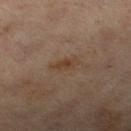This lesion was catalogued during total-body skin photography and was not selected for biopsy.
The lesion-visualizer software estimated a lesion color around L≈37 a*≈15 b*≈27 in CIELAB, a lesion–skin lightness drop of about 5, and a lesion-to-skin contrast of about 6.5 (normalized; higher = more distinct). It also reported border irregularity of about 4 on a 0–10 scale, a within-lesion color-variation index near 3/10, and peripheral color asymmetry of about 1. And it measured a nevus-likeness score of about 10/100 and a detector confidence of about 100 out of 100 that the crop contains a lesion.
Cropped from a total-body skin-imaging series; the visible field is about 15 mm.
Located on the right thigh.
A male subject aged 58 to 62.
This is a cross-polarized tile.
Longest diameter approximately 3.5 mm.